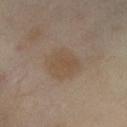{
  "biopsy_status": "not biopsied; imaged during a skin examination",
  "image": {
    "source": "total-body photography crop",
    "field_of_view_mm": 15
  },
  "automated_metrics": {
    "cielab_L": 50,
    "cielab_a": 13,
    "cielab_b": 28,
    "vs_skin_contrast_norm": 5.5,
    "border_irregularity_0_10": 2.5,
    "color_variation_0_10": 2.0,
    "peripheral_color_asymmetry": 0.5,
    "nevus_likeness_0_100": 5,
    "lesion_detection_confidence_0_100": 100
  },
  "patient": {
    "sex": "female",
    "age_approx": 55
  },
  "lesion_size": {
    "long_diameter_mm_approx": 4.5
  },
  "site": "abdomen",
  "lighting": "cross-polarized"
}